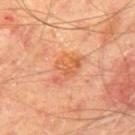No biopsy was performed on this lesion — it was imaged during a full skin examination and was not determined to be concerning. From the mid back. This image is a 15 mm lesion crop taken from a total-body photograph. The patient is a male roughly 65 years of age. Measured at roughly 4.5 mm in maximum diameter.